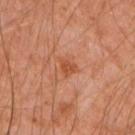Clinical impression: The lesion was photographed on a routine skin check and not biopsied; there is no pathology result. Context: About 2 mm across. A region of skin cropped from a whole-body photographic capture, roughly 15 mm wide. A male patient, about 45 years old. From the arm. Imaged with cross-polarized lighting.